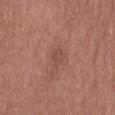Q: What did automated image analysis measure?
A: a detector confidence of about 100 out of 100 that the crop contains a lesion
Q: How was the tile lit?
A: white-light
Q: Where on the body is the lesion?
A: the leg
Q: What is the imaging modality?
A: ~15 mm tile from a whole-body skin photo
Q: Patient demographics?
A: female, in their mid- to late 60s
Q: Lesion size?
A: about 2.5 mm